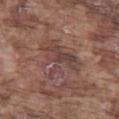Imaged during a routine full-body skin examination; the lesion was not biopsied and no histopathology is available. A close-up tile cropped from a whole-body skin photograph, about 15 mm across. Approximately 6 mm at its widest. A male subject in their mid- to late 70s. Automated tile analysis of the lesion measured an area of roughly 17 mm², a shape eccentricity near 0.65, and a symmetry-axis asymmetry near 0.55. The software also gave a border-irregularity index near 9/10, a color-variation rating of about 4.5/10, and radial color variation of about 1.5. It also reported lesion-presence confidence of about 95/100. The tile uses white-light illumination. The lesion is located on the left thigh.